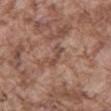From the back. Cropped from a whole-body photographic skin survey; the tile spans about 15 mm. The patient is a male aged around 75.